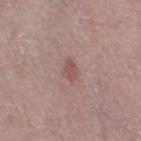workup = no biopsy performed (imaged during a skin exam); location = the right thigh; patient = female, aged 48 to 52; imaging modality = ~15 mm crop, total-body skin-cancer survey; illumination = white-light; diameter = ~2.5 mm (longest diameter).